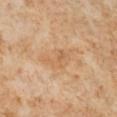Image and clinical context: On the leg. A roughly 15 mm field-of-view crop from a total-body skin photograph. A female subject, aged approximately 50.